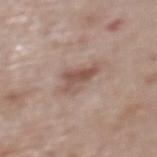Recorded during total-body skin imaging; not selected for excision or biopsy. Captured under white-light illumination. A 15 mm close-up extracted from a 3D total-body photography capture. Longest diameter approximately 4 mm. A female patient, about 65 years old. Automated tile analysis of the lesion measured a footprint of about 7 mm², an eccentricity of roughly 0.75, and a shape-asymmetry score of about 0.5 (0 = symmetric). The analysis additionally found border irregularity of about 5 on a 0–10 scale, a within-lesion color-variation index near 4.5/10, and peripheral color asymmetry of about 1.5. The analysis additionally found an automated nevus-likeness rating near 10 out of 100. On the upper back.